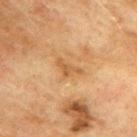Imaged during a routine full-body skin examination; the lesion was not biopsied and no histopathology is available.
On the back.
A 15 mm close-up extracted from a 3D total-body photography capture.
The tile uses cross-polarized illumination.
The patient is a male aged approximately 75.
Measured at roughly 3 mm in maximum diameter.